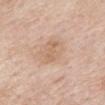Image and clinical context: The patient is a male in their mid- to late 80s or older. Captured under white-light illumination. A lesion tile, about 15 mm wide, cut from a 3D total-body photograph. Located on the back. About 3 mm across. An algorithmic analysis of the crop reported an area of roughly 3 mm², a shape eccentricity near 0.9, and a symmetry-axis asymmetry near 0.55. It also reported a lesion color around L≈63 a*≈19 b*≈32 in CIELAB and about 7 CIELAB-L* units darker than the surrounding skin. It also reported a border-irregularity rating of about 6.5/10, a within-lesion color-variation index near 0/10, and peripheral color asymmetry of about 0. And it measured a nevus-likeness score of about 0/100.